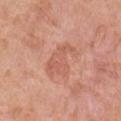biopsy_status: not biopsied; imaged during a skin examination
lesion_size:
  long_diameter_mm_approx: 4.5
lighting: white-light
patient:
  sex: male
  age_approx: 55
site: right upper arm
image:
  source: total-body photography crop
  field_of_view_mm: 15